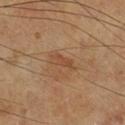Part of a total-body skin-imaging series; this lesion was reviewed on a skin check and was not flagged for biopsy. The lesion's longest dimension is about 3.5 mm. A male patient aged 63 to 67. From the right lower leg. The tile uses cross-polarized illumination. The total-body-photography lesion software estimated a footprint of about 4 mm², an outline eccentricity of about 0.9 (0 = round, 1 = elongated), and two-axis asymmetry of about 0.3. It also reported a lesion color around L≈48 a*≈21 b*≈33 in CIELAB and a normalized lesion–skin contrast near 5.5. And it measured border irregularity of about 3.5 on a 0–10 scale, a within-lesion color-variation index near 1.5/10, and peripheral color asymmetry of about 0.5. And it measured a classifier nevus-likeness of about 0/100 and a lesion-detection confidence of about 100/100. A roughly 15 mm field-of-view crop from a total-body skin photograph.